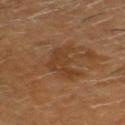Assessment:
No biopsy was performed on this lesion — it was imaged during a full skin examination and was not determined to be concerning.
Background:
A male patient, aged around 60. A 15 mm close-up extracted from a 3D total-body photography capture. Measured at roughly 4.5 mm in maximum diameter. Automated image analysis of the tile measured a shape eccentricity near 0.8 and two-axis asymmetry of about 0.55. The software also gave a mean CIELAB color near L≈36 a*≈19 b*≈32, roughly 6 lightness units darker than nearby skin, and a normalized border contrast of about 6. And it measured an automated nevus-likeness rating near 0 out of 100 and lesion-presence confidence of about 100/100. Located on the head or neck. The tile uses cross-polarized illumination.